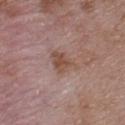| feature | finding |
|---|---|
| notes | imaged on a skin check; not biopsied |
| lesion size | about 4 mm |
| site | the upper back |
| automated lesion analysis | a lesion area of about 7.5 mm² and a shape-asymmetry score of about 0.35 (0 = symmetric); a lesion color around L≈50 a*≈18 b*≈25 in CIELAB, roughly 8 lightness units darker than nearby skin, and a normalized lesion–skin contrast near 7; a border-irregularity index near 3.5/10 and internal color variation of about 3.5 on a 0–10 scale; a classifier nevus-likeness of about 0/100 |
| subject | male, about 50 years old |
| image source | total-body-photography crop, ~15 mm field of view |
| illumination | white-light |